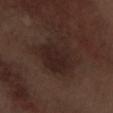Q: Is there a histopathology result?
A: total-body-photography surveillance lesion; no biopsy
Q: What is the anatomic site?
A: the left forearm
Q: What is the imaging modality?
A: ~15 mm crop, total-body skin-cancer survey
Q: Patient demographics?
A: male, roughly 70 years of age